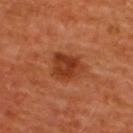<lesion>
<biopsy_status>not biopsied; imaged during a skin examination</biopsy_status>
<site>upper back</site>
<lighting>cross-polarized</lighting>
<lesion_size>
  <long_diameter_mm_approx>4.0</long_diameter_mm_approx>
</lesion_size>
<automated_metrics>
  <eccentricity>0.55</eccentricity>
  <shape_asymmetry>0.25</shape_asymmetry>
  <vs_skin_darker_L>10.0</vs_skin_darker_L>
  <vs_skin_contrast_norm>8.5</vs_skin_contrast_norm>
  <color_variation_0_10>4.5</color_variation_0_10>
  <peripheral_color_asymmetry>1.5</peripheral_color_asymmetry>
  <nevus_likeness_0_100>90</nevus_likeness_0_100>
</automated_metrics>
<image>
  <source>total-body photography crop</source>
  <field_of_view_mm>15</field_of_view_mm>
</image>
<patient>
  <sex>female</sex>
  <age_approx>45</age_approx>
</patient>
</lesion>